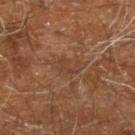{"biopsy_status": "not biopsied; imaged during a skin examination", "lighting": "cross-polarized", "image": {"source": "total-body photography crop", "field_of_view_mm": 15}, "automated_metrics": {"area_mm2_approx": 3.0, "eccentricity": 0.85, "shape_asymmetry": 0.55, "vs_skin_darker_L": 5.0, "vs_skin_contrast_norm": 5.0, "color_variation_0_10": 0.5, "peripheral_color_asymmetry": 0.0}, "lesion_size": {"long_diameter_mm_approx": 3.0}, "patient": {"sex": "male", "age_approx": 60}, "site": "right leg"}